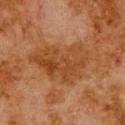biopsy status=no biopsy performed (imaged during a skin exam); image=~15 mm tile from a whole-body skin photo; tile lighting=cross-polarized; patient=male, aged around 80; diameter=≈7.5 mm; automated metrics=a lesion area of about 24 mm² and an eccentricity of roughly 0.75; site=the upper back.